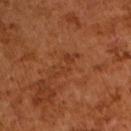Captured during whole-body skin photography for melanoma surveillance; the lesion was not biopsied. Imaged with cross-polarized lighting. A male subject, aged 63 to 67. A close-up tile cropped from a whole-body skin photograph, about 15 mm across. Automated tile analysis of the lesion measured two-axis asymmetry of about 0.65. Measured at roughly 4.5 mm in maximum diameter.